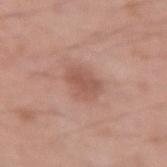The lesion was photographed on a routine skin check and not biopsied; there is no pathology result.
Imaged with white-light lighting.
A lesion tile, about 15 mm wide, cut from a 3D total-body photograph.
A male subject about 20 years old.
Located on the arm.